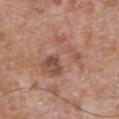Clinical impression: The lesion was tiled from a total-body skin photograph and was not biopsied. Image and clinical context: From the front of the torso. Automated tile analysis of the lesion measured a lesion color around L≈53 a*≈21 b*≈28 in CIELAB, about 7 CIELAB-L* units darker than the surrounding skin, and a normalized lesion–skin contrast near 5.5. It also reported a nevus-likeness score of about 0/100. The tile uses white-light illumination. A male patient in their mid- to late 60s. About 6 mm across. Cropped from a total-body skin-imaging series; the visible field is about 15 mm.